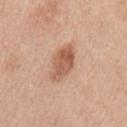notes = imaged on a skin check; not biopsied | illumination = white-light illumination | subject = female, aged 43–47 | imaging modality = 15 mm crop, total-body photography | diameter = about 4.5 mm | location = the left upper arm.